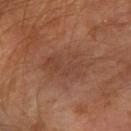{
  "biopsy_status": "not biopsied; imaged during a skin examination",
  "image": {
    "source": "total-body photography crop",
    "field_of_view_mm": 15
  },
  "lesion_size": {
    "long_diameter_mm_approx": 5.0
  },
  "lighting": "cross-polarized",
  "patient": {
    "sex": "male",
    "age_approx": 60
  },
  "site": "left forearm"
}